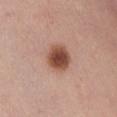  biopsy_status: not biopsied; imaged during a skin examination
  lighting: white-light
  image:
    source: total-body photography crop
    field_of_view_mm: 15
  patient:
    sex: male
    age_approx: 55
  automated_metrics:
    area_mm2_approx: 9.5
    eccentricity: 0.35
    border_irregularity_0_10: 1.5
    color_variation_0_10: 4.5
    nevus_likeness_0_100: 100
    lesion_detection_confidence_0_100: 100
  lesion_size:
    long_diameter_mm_approx: 3.5
  site: arm